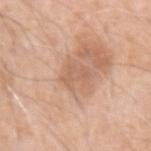workup = total-body-photography surveillance lesion; no biopsy | imaging modality = 15 mm crop, total-body photography | subject = male, roughly 60 years of age | tile lighting = white-light illumination | anatomic site = the left upper arm.